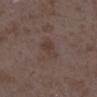Notes:
• notes: total-body-photography surveillance lesion; no biopsy
• lesion size: ~3 mm (longest diameter)
• patient: female, roughly 35 years of age
• tile lighting: white-light illumination
• location: the leg
• image source: ~15 mm tile from a whole-body skin photo